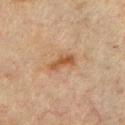Recorded during total-body skin imaging; not selected for excision or biopsy.